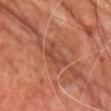– follow-up: total-body-photography surveillance lesion; no biopsy
– body site: the chest
– TBP lesion metrics: roughly 7 lightness units darker than nearby skin and a lesion-to-skin contrast of about 5.5 (normalized; higher = more distinct); a lesion-detection confidence of about 60/100
– lesion diameter: about 4 mm
– image: ~15 mm crop, total-body skin-cancer survey
– illumination: cross-polarized illumination
– patient: male, approximately 65 years of age